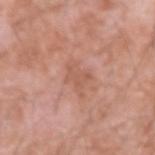Q: Was this lesion biopsied?
A: total-body-photography surveillance lesion; no biopsy
Q: How was the tile lit?
A: white-light illumination
Q: Where on the body is the lesion?
A: the arm
Q: Who is the patient?
A: male, aged 58–62
Q: How was this image acquired?
A: ~15 mm crop, total-body skin-cancer survey
Q: How large is the lesion?
A: ≈3 mm